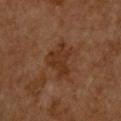Notes:
• biopsy status: no biopsy performed (imaged during a skin exam)
• body site: the upper back
• patient: male, roughly 60 years of age
• diameter: ≈4.5 mm
• acquisition: total-body-photography crop, ~15 mm field of view